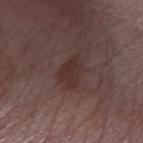The lesion was tiled from a total-body skin photograph and was not biopsied.
From the right forearm.
A male patient aged 73 to 77.
About 3.5 mm across.
Cropped from a total-body skin-imaging series; the visible field is about 15 mm.
This is a white-light tile.